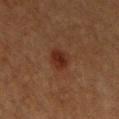| field | value |
|---|---|
| notes | catalogued during a skin exam; not biopsied |
| acquisition | ~15 mm tile from a whole-body skin photo |
| automated lesion analysis | an area of roughly 4.5 mm², an outline eccentricity of about 0.55 (0 = round, 1 = elongated), and two-axis asymmetry of about 0.15; a border-irregularity rating of about 1.5/10, a color-variation rating of about 2/10, and a peripheral color-asymmetry measure near 0.5 |
| subject | female, aged 38–42 |
| illumination | cross-polarized |
| location | the chest |